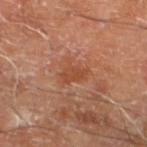The lesion was photographed on a routine skin check and not biopsied; there is no pathology result. The tile uses cross-polarized illumination. A male subject approximately 70 years of age. The lesion is located on the left lower leg. A region of skin cropped from a whole-body photographic capture, roughly 15 mm wide. Longest diameter approximately 3.5 mm.